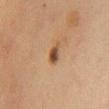Notes:
* biopsy status — imaged on a skin check; not biopsied
* subject — female, aged around 50
* acquisition — ~15 mm tile from a whole-body skin photo
* illumination — cross-polarized
* lesion size — ~2.5 mm (longest diameter)
* location — the front of the torso